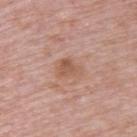<record>
  <biopsy_status>not biopsied; imaged during a skin examination</biopsy_status>
  <site>upper back</site>
  <patient>
    <sex>male</sex>
    <age_approx>65</age_approx>
  </patient>
  <image>
    <source>total-body photography crop</source>
    <field_of_view_mm>15</field_of_view_mm>
  </image>
  <lighting>white-light</lighting>
  <automated_metrics>
    <area_mm2_approx>5.5</area_mm2_approx>
    <eccentricity>0.7</eccentricity>
    <shape_asymmetry>0.4</shape_asymmetry>
    <vs_skin_darker_L>8.0</vs_skin_darker_L>
    <vs_skin_contrast_norm>6.0</vs_skin_contrast_norm>
  </automated_metrics>
</record>